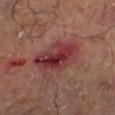Clinical impression:
This lesion was catalogued during total-body skin photography and was not selected for biopsy.
Clinical summary:
The lesion is located on the right thigh. A roughly 15 mm field-of-view crop from a total-body skin photograph.